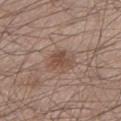Findings:
- workup · imaged on a skin check; not biopsied
- patient · male, aged approximately 60
- imaging modality · ~15 mm tile from a whole-body skin photo
- anatomic site · the left lower leg